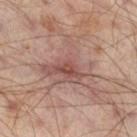biopsy status: total-body-photography surveillance lesion; no biopsy
image-analysis metrics: a border-irregularity index near 7/10, a within-lesion color-variation index near 6.5/10, and radial color variation of about 2; a detector confidence of about 80 out of 100 that the crop contains a lesion
anatomic site: the right thigh
lesion diameter: about 6 mm
patient: male, aged 63–67
image source: total-body-photography crop, ~15 mm field of view